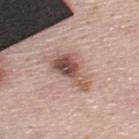Assessment: Part of a total-body skin-imaging series; this lesion was reviewed on a skin check and was not flagged for biopsy. Background: On the upper back. Longest diameter approximately 5.5 mm. A male patient, in their mid- to late 40s. Automated image analysis of the tile measured a lesion color around L≈53 a*≈19 b*≈24 in CIELAB, roughly 14 lightness units darker than nearby skin, and a normalized border contrast of about 9.5. It also reported a border-irregularity rating of about 5/10, a color-variation rating of about 8.5/10, and peripheral color asymmetry of about 3. The analysis additionally found an automated nevus-likeness rating near 50 out of 100. The tile uses white-light illumination. A 15 mm close-up tile from a total-body photography series done for melanoma screening.